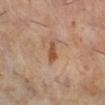automated lesion analysis: a lesion area of about 3 mm² and an outline eccentricity of about 0.85 (0 = round, 1 = elongated)
subject: female, aged around 60
size: ~2.5 mm (longest diameter)
imaging modality: 15 mm crop, total-body photography
site: the right lower leg